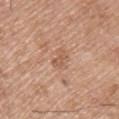Clinical impression:
Recorded during total-body skin imaging; not selected for excision or biopsy.
Context:
The lesion is located on the left upper arm. Automated image analysis of the tile measured an outline eccentricity of about 0.9 (0 = round, 1 = elongated) and a symmetry-axis asymmetry near 0.35. The analysis additionally found border irregularity of about 4 on a 0–10 scale and a peripheral color-asymmetry measure near 0. And it measured an automated nevus-likeness rating near 0 out of 100 and a detector confidence of about 100 out of 100 that the crop contains a lesion. About 2.5 mm across. A male subject, aged 63–67. A roughly 15 mm field-of-view crop from a total-body skin photograph. Captured under white-light illumination.